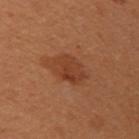Part of a total-body skin-imaging series; this lesion was reviewed on a skin check and was not flagged for biopsy.
A female patient, in their 50s.
A lesion tile, about 15 mm wide, cut from a 3D total-body photograph.
On the left upper arm.
The lesion's longest dimension is about 3.5 mm.
Automated tile analysis of the lesion measured an area of roughly 8.5 mm², an eccentricity of roughly 0.45, and two-axis asymmetry of about 0.15. The analysis additionally found border irregularity of about 1.5 on a 0–10 scale. And it measured a detector confidence of about 100 out of 100 that the crop contains a lesion.
This is a cross-polarized tile.